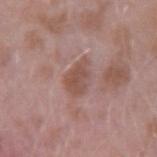<case>
  <biopsy_status>not biopsied; imaged during a skin examination</biopsy_status>
  <patient>
    <sex>male</sex>
    <age_approx>40</age_approx>
  </patient>
  <image>
    <source>total-body photography crop</source>
    <field_of_view_mm>15</field_of_view_mm>
  </image>
  <site>right upper arm</site>
</case>